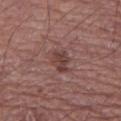The lesion was tiled from a total-body skin photograph and was not biopsied. A roughly 15 mm field-of-view crop from a total-body skin photograph. On the left lower leg. The subject is a male roughly 65 years of age. This is a white-light tile.